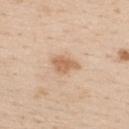Impression: This lesion was catalogued during total-body skin photography and was not selected for biopsy. Context: The tile uses white-light illumination. Automated tile analysis of the lesion measured a lesion color around L≈63 a*≈20 b*≈34 in CIELAB, about 11 CIELAB-L* units darker than the surrounding skin, and a normalized lesion–skin contrast near 7.5. The software also gave a border-irregularity index near 3/10, a color-variation rating of about 1.5/10, and a peripheral color-asymmetry measure near 0.5. It also reported a classifier nevus-likeness of about 50/100. About 3.5 mm across. A male subject, in their mid- to late 20s. On the upper back. Cropped from a total-body skin-imaging series; the visible field is about 15 mm.